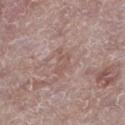The lesion was tiled from a total-body skin photograph and was not biopsied. The patient is a female aged 63–67. The lesion is on the left lower leg. The total-body-photography lesion software estimated roughly 6 lightness units darker than nearby skin and a lesion-to-skin contrast of about 5 (normalized; higher = more distinct). This is a white-light tile. A lesion tile, about 15 mm wide, cut from a 3D total-body photograph.